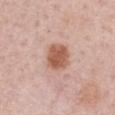From the right upper arm.
A 15 mm crop from a total-body photograph taken for skin-cancer surveillance.
The recorded lesion diameter is about 3.5 mm.
Imaged with white-light lighting.
A male subject, aged around 60.